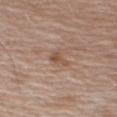Captured during whole-body skin photography for melanoma surveillance; the lesion was not biopsied. The tile uses white-light illumination. A male subject aged around 65. This image is a 15 mm lesion crop taken from a total-body photograph. On the right upper arm.